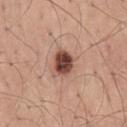  biopsy_status: not biopsied; imaged during a skin examination
  site: mid back
  patient:
    sex: male
    age_approx: 65
  image:
    source: total-body photography crop
    field_of_view_mm: 15
  automated_metrics:
    cielab_L: 47
    cielab_a: 23
    cielab_b: 27
    vs_skin_contrast_norm: 12.0
    border_irregularity_0_10: 2.0
    color_variation_0_10: 6.0
    peripheral_color_asymmetry: 2.0
  lighting: white-light
  lesion_size:
    long_diameter_mm_approx: 3.5